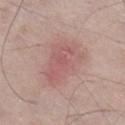Captured during whole-body skin photography for melanoma surveillance; the lesion was not biopsied. A 15 mm crop from a total-body photograph taken for skin-cancer surveillance. The lesion-visualizer software estimated a mean CIELAB color near L≈58 a*≈22 b*≈22 and a normalized lesion–skin contrast near 5. And it measured a peripheral color-asymmetry measure near 1. And it measured a nevus-likeness score of about 35/100 and a lesion-detection confidence of about 100/100. On the right lower leg. The subject is a male about 60 years old. The tile uses white-light illumination.